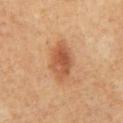site = the mid back
lesion size = ~5 mm (longest diameter)
patient = male, roughly 60 years of age
TBP lesion metrics = an automated nevus-likeness rating near 95 out of 100 and a lesion-detection confidence of about 100/100
illumination = cross-polarized illumination
image source = 15 mm crop, total-body photography